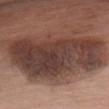  biopsy_status: not biopsied; imaged during a skin examination
  image:
    source: total-body photography crop
    field_of_view_mm: 15
  automated_metrics:
    cielab_L: 40
    cielab_a: 19
    cielab_b: 23
    vs_skin_darker_L: 16.0
    vs_skin_contrast_norm: 12.0
    border_irregularity_0_10: 5.0
    color_variation_0_10: 6.0
    peripheral_color_asymmetry: 2.0
    nevus_likeness_0_100: 0
    lesion_detection_confidence_0_100: 55
  lighting: white-light
  site: chest
  patient:
    sex: female
    age_approx: 75